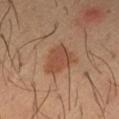Findings:
• notes · imaged on a skin check; not biopsied
• image · total-body-photography crop, ~15 mm field of view
• body site · the right forearm
• subject · male, approximately 40 years of age